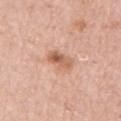The lesion was tiled from a total-body skin photograph and was not biopsied.
The lesion-visualizer software estimated a shape eccentricity near 0.8. The software also gave a color-variation rating of about 8/10 and a peripheral color-asymmetry measure near 3.5.
The subject is a female in their mid-60s.
This image is a 15 mm lesion crop taken from a total-body photograph.
Imaged with white-light lighting.
Located on the left upper arm.